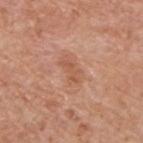The lesion was tiled from a total-body skin photograph and was not biopsied.
Measured at roughly 3 mm in maximum diameter.
A male patient, aged around 60.
Imaged with white-light lighting.
The total-body-photography lesion software estimated an average lesion color of about L≈55 a*≈24 b*≈33 (CIELAB), roughly 7 lightness units darker than nearby skin, and a normalized lesion–skin contrast near 5.5. And it measured an automated nevus-likeness rating near 0 out of 100 and a lesion-detection confidence of about 100/100.
The lesion is located on the back.
A region of skin cropped from a whole-body photographic capture, roughly 15 mm wide.